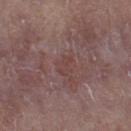notes: no biopsy performed (imaged during a skin exam)
patient: female, roughly 20 years of age
image source: ~15 mm tile from a whole-body skin photo
illumination: white-light illumination
anatomic site: the leg
TBP lesion metrics: a classifier nevus-likeness of about 0/100
lesion diameter: ≈2.5 mm